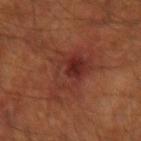Captured during whole-body skin photography for melanoma surveillance; the lesion was not biopsied. Cropped from a total-body skin-imaging series; the visible field is about 15 mm. On the right forearm. A male patient, approximately 60 years of age.